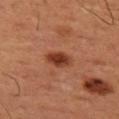follow-up = no biopsy performed (imaged during a skin exam)
patient = male, approximately 50 years of age
anatomic site = the left thigh
illumination = cross-polarized
image-analysis metrics = a border-irregularity rating of about 1.5/10, a color-variation rating of about 3.5/10, and radial color variation of about 1
imaging modality = ~15 mm tile from a whole-body skin photo